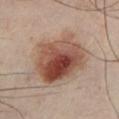Assessment:
No biopsy was performed on this lesion — it was imaged during a full skin examination and was not determined to be concerning.
Acquisition and patient details:
Automated tile analysis of the lesion measured a lesion area of about 28 mm², an eccentricity of roughly 0.55, and a shape-asymmetry score of about 0.15 (0 = symmetric). The software also gave an average lesion color of about L≈49 a*≈21 b*≈28 (CIELAB) and a normalized border contrast of about 10.5. A 15 mm close-up tile from a total-body photography series done for melanoma screening. A male subject roughly 40 years of age. The lesion's longest dimension is about 6.5 mm. On the left lower leg.